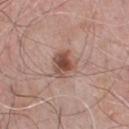Notes:
* biopsy status: no biopsy performed (imaged during a skin exam)
* automated metrics: lesion-presence confidence of about 100/100
* patient: male, approximately 65 years of age
* body site: the front of the torso
* image source: ~15 mm crop, total-body skin-cancer survey
* illumination: white-light illumination